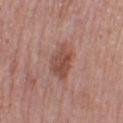biopsy_status: not biopsied; imaged during a skin examination
image:
  source: total-body photography crop
  field_of_view_mm: 15
automated_metrics:
  cielab_L: 49
  cielab_a: 23
  cielab_b: 26
  vs_skin_contrast_norm: 7.5
  border_irregularity_0_10: 3.0
  color_variation_0_10: 4.0
  peripheral_color_asymmetry: 1.5
  nevus_likeness_0_100: 25
  lesion_detection_confidence_0_100: 100
site: leg
patient:
  sex: female
  age_approx: 40
lighting: white-light
lesion_size:
  long_diameter_mm_approx: 4.5